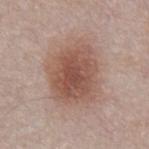<case>
  <biopsy_status>not biopsied; imaged during a skin examination</biopsy_status>
  <image>
    <source>total-body photography crop</source>
    <field_of_view_mm>15</field_of_view_mm>
  </image>
  <lesion_size>
    <long_diameter_mm_approx>6.5</long_diameter_mm_approx>
  </lesion_size>
  <patient>
    <sex>male</sex>
    <age_approx>55</age_approx>
  </patient>
  <site>abdomen</site>
</case>